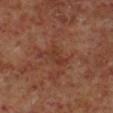Image and clinical context:
An algorithmic analysis of the crop reported a lesion color around L≈33 a*≈22 b*≈27 in CIELAB, a lesion–skin lightness drop of about 5, and a normalized lesion–skin contrast near 5.5. A 15 mm crop from a total-body photograph taken for skin-cancer surveillance. The lesion is on the right lower leg. A male patient, approximately 60 years of age.